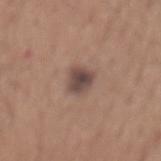Clinical summary: Captured under white-light illumination. This image is a 15 mm lesion crop taken from a total-body photograph. A male subject approximately 65 years of age. The lesion's longest dimension is about 3 mm. On the mid back.